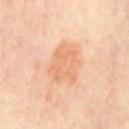Impression: No biopsy was performed on this lesion — it was imaged during a full skin examination and was not determined to be concerning. Acquisition and patient details: A patient aged 53–57. The total-body-photography lesion software estimated a shape eccentricity near 0.7 and a shape-asymmetry score of about 0.25 (0 = symmetric). The software also gave about 8 CIELAB-L* units darker than the surrounding skin and a normalized border contrast of about 5.5. And it measured an automated nevus-likeness rating near 20 out of 100. This is a cross-polarized tile. On the chest. Cropped from a whole-body photographic skin survey; the tile spans about 15 mm. The lesion's longest dimension is about 5 mm.